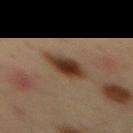{
  "biopsy_status": "not biopsied; imaged during a skin examination",
  "patient": {
    "sex": "male",
    "age_approx": 35
  },
  "image": {
    "source": "total-body photography crop",
    "field_of_view_mm": 15
  },
  "lesion_size": {
    "long_diameter_mm_approx": 4.5
  },
  "lighting": "cross-polarized",
  "site": "mid back",
  "automated_metrics": {
    "cielab_L": 33,
    "cielab_a": 15,
    "cielab_b": 26,
    "vs_skin_darker_L": 13.0,
    "border_irregularity_0_10": 2.0,
    "color_variation_0_10": 6.0,
    "nevus_likeness_0_100": 100,
    "lesion_detection_confidence_0_100": 100
  }
}